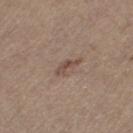workup: total-body-photography surveillance lesion; no biopsy
acquisition: ~15 mm crop, total-body skin-cancer survey
lesion size: ≈3 mm
patient: female, about 65 years old
image-analysis metrics: a footprint of about 2.5 mm², an eccentricity of roughly 0.9, and a shape-asymmetry score of about 0.5 (0 = symmetric); a lesion-to-skin contrast of about 7 (normalized; higher = more distinct); a classifier nevus-likeness of about 0/100 and a lesion-detection confidence of about 100/100
lighting: white-light illumination
anatomic site: the left thigh